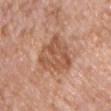{"biopsy_status": "not biopsied; imaged during a skin examination", "lesion_size": {"long_diameter_mm_approx": 6.0}, "lighting": "white-light", "patient": {"sex": "male", "age_approx": 75}, "site": "right upper arm", "automated_metrics": {"area_mm2_approx": 17.0, "cielab_L": 55, "cielab_a": 23, "cielab_b": 32, "vs_skin_darker_L": 10.0, "vs_skin_contrast_norm": 7.0, "border_irregularity_0_10": 3.5, "color_variation_0_10": 4.5, "peripheral_color_asymmetry": 1.5, "nevus_likeness_0_100": 5, "lesion_detection_confidence_0_100": 100}, "image": {"source": "total-body photography crop", "field_of_view_mm": 15}}